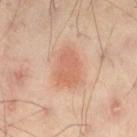notes — catalogued during a skin exam; not biopsied | acquisition — ~15 mm tile from a whole-body skin photo | tile lighting — cross-polarized | patient — male, aged 43–47 | location — the right thigh | size — ≈4 mm.